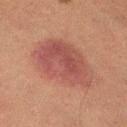Q: Was this lesion biopsied?
A: catalogued during a skin exam; not biopsied
Q: How was the tile lit?
A: cross-polarized
Q: Who is the patient?
A: female, in their mid- to late 50s
Q: What kind of image is this?
A: ~15 mm tile from a whole-body skin photo
Q: Lesion location?
A: the right lower leg
Q: Automated lesion metrics?
A: border irregularity of about 2.5 on a 0–10 scale, internal color variation of about 4 on a 0–10 scale, and peripheral color asymmetry of about 1.5
Q: What is the lesion's diameter?
A: about 7.5 mm